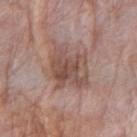Clinical summary: A female patient approximately 65 years of age. The total-body-photography lesion software estimated a footprint of about 17 mm² and two-axis asymmetry of about 0.25. The software also gave an average lesion color of about L≈50 a*≈18 b*≈24 (CIELAB), a lesion–skin lightness drop of about 10, and a normalized border contrast of about 7.5. The software also gave a border-irregularity rating of about 4/10, a color-variation rating of about 5/10, and peripheral color asymmetry of about 1.5. It also reported a classifier nevus-likeness of about 0/100 and lesion-presence confidence of about 100/100. Cropped from a whole-body photographic skin survey; the tile spans about 15 mm. The lesion is on the right forearm. Captured under white-light illumination.